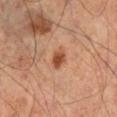Q: Is there a histopathology result?
A: total-body-photography surveillance lesion; no biopsy
Q: Who is the patient?
A: male, aged approximately 55
Q: Illumination type?
A: cross-polarized
Q: What did automated image analysis measure?
A: a mean CIELAB color near L≈50 a*≈26 b*≈35 and a lesion–skin lightness drop of about 12; border irregularity of about 2 on a 0–10 scale, a color-variation rating of about 3/10, and a peripheral color-asymmetry measure near 1; a classifier nevus-likeness of about 95/100 and lesion-presence confidence of about 100/100
Q: Lesion location?
A: the right lower leg
Q: What is the imaging modality?
A: ~15 mm crop, total-body skin-cancer survey
Q: How large is the lesion?
A: ≈2.5 mm